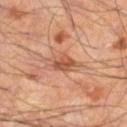Clinical impression: Part of a total-body skin-imaging series; this lesion was reviewed on a skin check and was not flagged for biopsy.